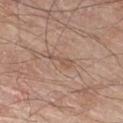{
  "biopsy_status": "not biopsied; imaged during a skin examination",
  "lighting": "white-light",
  "image": {
    "source": "total-body photography crop",
    "field_of_view_mm": 15
  },
  "site": "leg",
  "patient": {
    "sex": "male",
    "age_approx": 80
  },
  "lesion_size": {
    "long_diameter_mm_approx": 3.0
  }
}